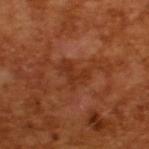notes: catalogued during a skin exam; not biopsied | patient: male, approximately 65 years of age | image: ~15 mm tile from a whole-body skin photo | size: about 3 mm | lighting: cross-polarized.